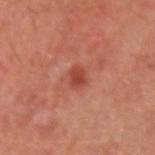biopsy status: catalogued during a skin exam; not biopsied | automated metrics: a lesion color around L≈44 a*≈31 b*≈31 in CIELAB, roughly 9 lightness units darker than nearby skin, and a normalized border contrast of about 7; a border-irregularity rating of about 3/10, a color-variation rating of about 2/10, and peripheral color asymmetry of about 0.5; a nevus-likeness score of about 40/100 and a detector confidence of about 100 out of 100 that the crop contains a lesion | anatomic site: the left upper arm | patient: male, aged 53 to 57 | lesion diameter: about 2.5 mm | lighting: cross-polarized | acquisition: total-body-photography crop, ~15 mm field of view.